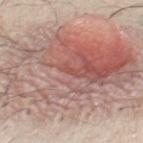follow-up: no biopsy performed (imaged during a skin exam) | acquisition: total-body-photography crop, ~15 mm field of view | anatomic site: the front of the torso | subject: male, aged around 60.